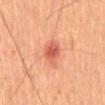Q: Was a biopsy performed?
A: no biopsy performed (imaged during a skin exam)
Q: Where on the body is the lesion?
A: the abdomen
Q: What is the lesion's diameter?
A: about 3 mm
Q: What is the imaging modality?
A: total-body-photography crop, ~15 mm field of view
Q: Who is the patient?
A: male, aged approximately 55